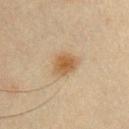image:
  source: total-body photography crop
  field_of_view_mm: 15
site: front of the torso
lesion_size:
  long_diameter_mm_approx: 3.5
patient:
  sex: male
  age_approx: 35
automated_metrics:
  area_mm2_approx: 7.0
  eccentricity: 0.65
  shape_asymmetry: 0.25
  color_variation_0_10: 3.0
  nevus_likeness_0_100: 100
  lesion_detection_confidence_0_100: 100